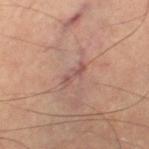Part of a total-body skin-imaging series; this lesion was reviewed on a skin check and was not flagged for biopsy. A male patient aged approximately 65. The lesion is located on the right thigh. Measured at roughly 3 mm in maximum diameter. Imaged with cross-polarized lighting. A 15 mm close-up extracted from a 3D total-body photography capture.